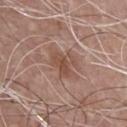Recorded during total-body skin imaging; not selected for excision or biopsy. Located on the chest. The lesion's longest dimension is about 4 mm. A roughly 15 mm field-of-view crop from a total-body skin photograph. The patient is a male about 65 years old.